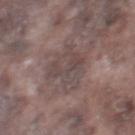biopsy status=catalogued during a skin exam; not biopsied
lesion diameter=about 5.5 mm
image source=~15 mm tile from a whole-body skin photo
anatomic site=the right thigh
subject=male, approximately 75 years of age